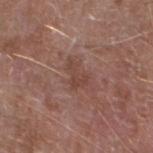Impression:
Captured during whole-body skin photography for melanoma surveillance; the lesion was not biopsied.
Clinical summary:
The tile uses white-light illumination. The lesion is located on the left lower leg. A male patient, approximately 65 years of age. Automated image analysis of the tile measured a footprint of about 3.5 mm² and two-axis asymmetry of about 0.65. It also reported border irregularity of about 7.5 on a 0–10 scale, internal color variation of about 1 on a 0–10 scale, and peripheral color asymmetry of about 0. Cropped from a whole-body photographic skin survey; the tile spans about 15 mm. About 3 mm across.